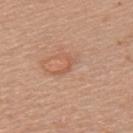Assessment: No biopsy was performed on this lesion — it was imaged during a full skin examination and was not determined to be concerning. Acquisition and patient details: The lesion is on the upper back. Cropped from a whole-body photographic skin survey; the tile spans about 15 mm. The subject is a female roughly 55 years of age. This is a white-light tile.